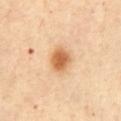notes = imaged on a skin check; not biopsied